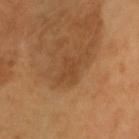The lesion was tiled from a total-body skin photograph and was not biopsied. A male patient roughly 45 years of age. On the head or neck. Automated image analysis of the tile measured a lesion area of about 4.5 mm², an outline eccentricity of about 0.65 (0 = round, 1 = elongated), and a symmetry-axis asymmetry near 0.35. And it measured a lesion–skin lightness drop of about 6 and a normalized lesion–skin contrast near 4.5. The analysis additionally found a border-irregularity index near 4/10, internal color variation of about 1.5 on a 0–10 scale, and a peripheral color-asymmetry measure near 0.5. A lesion tile, about 15 mm wide, cut from a 3D total-body photograph. The recorded lesion diameter is about 3 mm.